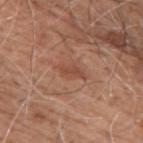follow-up — imaged on a skin check; not biopsied
image-analysis metrics — an outline eccentricity of about 0.9 (0 = round, 1 = elongated) and a shape-asymmetry score of about 0.35 (0 = symmetric); border irregularity of about 4 on a 0–10 scale and a within-lesion color-variation index near 1.5/10; a nevus-likeness score of about 0/100 and a lesion-detection confidence of about 100/100
lighting — white-light illumination
site — the back
image source — total-body-photography crop, ~15 mm field of view
subject — male, roughly 60 years of age
diameter — ≈3.5 mm